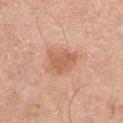biopsy status=catalogued during a skin exam; not biopsied | lighting=white-light | subject=female, aged approximately 50 | anatomic site=the right upper arm | lesion size=~4 mm (longest diameter) | image=~15 mm tile from a whole-body skin photo.